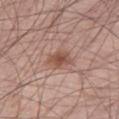Q: Patient demographics?
A: male, in their mid-60s
Q: What did automated image analysis measure?
A: a lesion color around L≈52 a*≈21 b*≈26 in CIELAB, roughly 10 lightness units darker than nearby skin, and a normalized border contrast of about 7.5; border irregularity of about 2.5 on a 0–10 scale and internal color variation of about 4 on a 0–10 scale
Q: What is the imaging modality?
A: 15 mm crop, total-body photography
Q: Where on the body is the lesion?
A: the leg